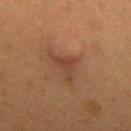Findings:
* notes — imaged on a skin check; not biopsied
* acquisition — ~15 mm tile from a whole-body skin photo
* illumination — cross-polarized
* automated lesion analysis — a lesion-detection confidence of about 95/100
* lesion size — about 4 mm
* patient — female, in their 50s
* site — the mid back